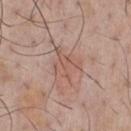Captured during whole-body skin photography for melanoma surveillance; the lesion was not biopsied.
The total-body-photography lesion software estimated an area of roughly 9.5 mm² and two-axis asymmetry of about 0.4. And it measured a border-irregularity index near 6/10, a color-variation rating of about 3/10, and a peripheral color-asymmetry measure near 1.5. And it measured a nevus-likeness score of about 0/100 and a lesion-detection confidence of about 100/100.
About 4.5 mm across.
From the chest.
A male patient aged approximately 45.
Cropped from a whole-body photographic skin survey; the tile spans about 15 mm.